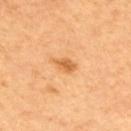| key | value |
|---|---|
| workup | no biopsy performed (imaged during a skin exam) |
| anatomic site | the back |
| patient | male, in their mid- to late 60s |
| automated metrics | border irregularity of about 3 on a 0–10 scale, internal color variation of about 1 on a 0–10 scale, and peripheral color asymmetry of about 0.5; lesion-presence confidence of about 100/100 |
| image | total-body-photography crop, ~15 mm field of view |